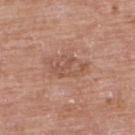The lesion was photographed on a routine skin check and not biopsied; there is no pathology result. From the upper back. Cropped from a whole-body photographic skin survey; the tile spans about 15 mm. Imaged with white-light lighting. A female patient roughly 60 years of age. The lesion-visualizer software estimated an area of roughly 8.5 mm², a shape eccentricity near 0.8, and a shape-asymmetry score of about 0.3 (0 = symmetric). The software also gave internal color variation of about 4 on a 0–10 scale and a peripheral color-asymmetry measure near 1. It also reported an automated nevus-likeness rating near 0 out of 100.